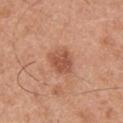workup: imaged on a skin check; not biopsied | imaging modality: total-body-photography crop, ~15 mm field of view | tile lighting: white-light illumination | subject: male, approximately 30 years of age | location: the upper back.